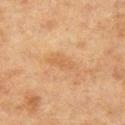Image and clinical context:
A female patient aged 38 to 42. Cropped from a whole-body photographic skin survey; the tile spans about 15 mm. From the left thigh. Approximately 4 mm at its widest. Automated tile analysis of the lesion measured border irregularity of about 3 on a 0–10 scale and a peripheral color-asymmetry measure near 0.5.